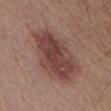subject: male, approximately 55 years of age
tile lighting: white-light illumination
size: ≈7.5 mm
acquisition: ~15 mm tile from a whole-body skin photo
anatomic site: the abdomen
automated lesion analysis: a lesion color around L≈43 a*≈21 b*≈22 in CIELAB, roughly 11 lightness units darker than nearby skin, and a normalized border contrast of about 8.5; an automated nevus-likeness rating near 45 out of 100 and a lesion-detection confidence of about 100/100